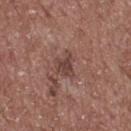Notes:
* follow-up · total-body-photography surveillance lesion; no biopsy
* body site · the upper back
* size · ~3 mm (longest diameter)
* tile lighting · white-light
* automated lesion analysis · a lesion area of about 4.5 mm²; a mean CIELAB color near L≈42 a*≈20 b*≈23, a lesion–skin lightness drop of about 9, and a lesion-to-skin contrast of about 7.5 (normalized; higher = more distinct); a border-irregularity index near 4.5/10, a color-variation rating of about 1.5/10, and a peripheral color-asymmetry measure near 0.5
* image source · 15 mm crop, total-body photography
* subject · male, aged 58–62